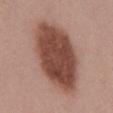This is a white-light tile. Located on the abdomen. The subject is a male aged approximately 40. About 10.5 mm across. Cropped from a total-body skin-imaging series; the visible field is about 15 mm.